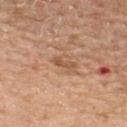<tbp_lesion>
  <biopsy_status>not biopsied; imaged during a skin examination</biopsy_status>
  <lesion_size>
    <long_diameter_mm_approx>3.0</long_diameter_mm_approx>
  </lesion_size>
  <patient>
    <sex>male</sex>
    <age_approx>60</age_approx>
  </patient>
  <site>upper back</site>
  <automated_metrics>
    <cielab_L>54</cielab_L>
    <cielab_a>22</cielab_a>
    <cielab_b>34</cielab_b>
    <vs_skin_darker_L>9.0</vs_skin_darker_L>
    <vs_skin_contrast_norm>6.5</vs_skin_contrast_norm>
    <nevus_likeness_0_100>0</nevus_likeness_0_100>
    <lesion_detection_confidence_0_100>100</lesion_detection_confidence_0_100>
  </automated_metrics>
  <lighting>white-light</lighting>
  <image>
    <source>total-body photography crop</source>
    <field_of_view_mm>15</field_of_view_mm>
  </image>
</tbp_lesion>